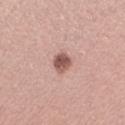Part of a total-body skin-imaging series; this lesion was reviewed on a skin check and was not flagged for biopsy. From the arm. A 15 mm crop from a total-body photograph taken for skin-cancer surveillance. Captured under white-light illumination. The lesion-visualizer software estimated a footprint of about 4.5 mm². The software also gave border irregularity of about 1.5 on a 0–10 scale and radial color variation of about 1.5. The software also gave a classifier nevus-likeness of about 85/100 and a lesion-detection confidence of about 100/100. Measured at roughly 2.5 mm in maximum diameter. The subject is a female about 35 years old.